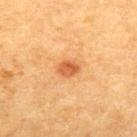biopsy_status: not biopsied; imaged during a skin examination
site: back
image:
  source: total-body photography crop
  field_of_view_mm: 15
patient:
  sex: male
  age_approx: 50
lighting: cross-polarized
lesion_size:
  long_diameter_mm_approx: 2.5